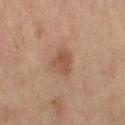No biopsy was performed on this lesion — it was imaged during a full skin examination and was not determined to be concerning.
Imaged with cross-polarized lighting.
The lesion is located on the right thigh.
This image is a 15 mm lesion crop taken from a total-body photograph.
A female patient, about 60 years old.